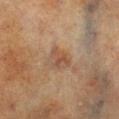Recorded during total-body skin imaging; not selected for excision or biopsy.
The lesion is on the left lower leg.
Automated tile analysis of the lesion measured an eccentricity of roughly 0.75 and a shape-asymmetry score of about 0.4 (0 = symmetric). The analysis additionally found about 7 CIELAB-L* units darker than the surrounding skin and a normalized lesion–skin contrast near 6. And it measured border irregularity of about 4 on a 0–10 scale, internal color variation of about 2.5 on a 0–10 scale, and a peripheral color-asymmetry measure near 0.5.
The subject is a male aged around 70.
This is a cross-polarized tile.
A roughly 15 mm field-of-view crop from a total-body skin photograph.
Measured at roughly 3 mm in maximum diameter.